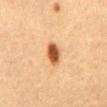Q: Was this lesion biopsied?
A: catalogued during a skin exam; not biopsied
Q: What is the anatomic site?
A: the front of the torso
Q: What is the imaging modality?
A: 15 mm crop, total-body photography
Q: Who is the patient?
A: male, approximately 50 years of age
Q: What is the lesion's diameter?
A: about 3 mm